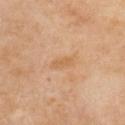follow-up: no biopsy performed (imaged during a skin exam) | illumination: cross-polarized | anatomic site: the chest | patient: female, about 50 years old | automated lesion analysis: an area of roughly 3 mm² and a shape-asymmetry score of about 0.3 (0 = symmetric); roughly 6 lightness units darker than nearby skin and a normalized border contrast of about 5.5; border irregularity of about 3 on a 0–10 scale and a color-variation rating of about 0/10; a classifier nevus-likeness of about 0/100 and lesion-presence confidence of about 100/100 | acquisition: ~15 mm tile from a whole-body skin photo.